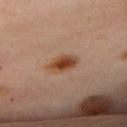Q: Was a biopsy performed?
A: total-body-photography surveillance lesion; no biopsy
Q: Who is the patient?
A: female, approximately 45 years of age
Q: What is the imaging modality?
A: ~15 mm crop, total-body skin-cancer survey
Q: What lighting was used for the tile?
A: cross-polarized illumination
Q: How large is the lesion?
A: ≈3.5 mm
Q: Where on the body is the lesion?
A: the chest